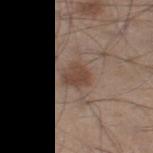Imaged during a routine full-body skin examination; the lesion was not biopsied and no histopathology is available.
A male subject aged approximately 45.
The lesion is located on the leg.
This is a white-light tile.
A region of skin cropped from a whole-body photographic capture, roughly 15 mm wide.
Approximately 2.5 mm at its widest.